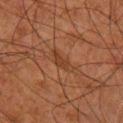Assessment:
The lesion was tiled from a total-body skin photograph and was not biopsied.
Clinical summary:
The lesion's longest dimension is about 3.5 mm. The lesion is on the leg. A male subject, approximately 80 years of age. A region of skin cropped from a whole-body photographic capture, roughly 15 mm wide.